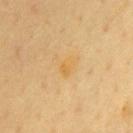Q: Was this lesion biopsied?
A: imaged on a skin check; not biopsied
Q: How large is the lesion?
A: ~2.5 mm (longest diameter)
Q: Who is the patient?
A: male, in their mid-60s
Q: What is the anatomic site?
A: the chest
Q: How was this image acquired?
A: ~15 mm crop, total-body skin-cancer survey
Q: Automated lesion metrics?
A: a border-irregularity index near 3.5/10, a within-lesion color-variation index near 2/10, and peripheral color asymmetry of about 0.5